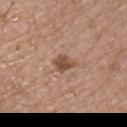workup: total-body-photography surveillance lesion; no biopsy
tile lighting: white-light illumination
automated lesion analysis: an eccentricity of roughly 0.65; a mean CIELAB color near L≈50 a*≈20 b*≈29, a lesion–skin lightness drop of about 11, and a lesion-to-skin contrast of about 8.5 (normalized; higher = more distinct); a border-irregularity index near 2.5/10
image source: ~15 mm tile from a whole-body skin photo
site: the right upper arm
subject: male, aged 53–57